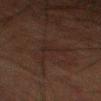Assessment: No biopsy was performed on this lesion — it was imaged during a full skin examination and was not determined to be concerning. Image and clinical context: A 15 mm crop from a total-body photograph taken for skin-cancer surveillance. From the leg. The lesion's longest dimension is about 6 mm. A male patient, in their 80s. An algorithmic analysis of the crop reported an area of roughly 7 mm², an outline eccentricity of about 0.95 (0 = round, 1 = elongated), and a symmetry-axis asymmetry near 0.75. The analysis additionally found a within-lesion color-variation index near 2/10 and a peripheral color-asymmetry measure near 0.5.